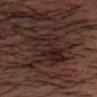<case>
  <biopsy_status>not biopsied; imaged during a skin examination</biopsy_status>
  <lighting>cross-polarized</lighting>
  <lesion_size>
    <long_diameter_mm_approx>6.0</long_diameter_mm_approx>
  </lesion_size>
  <patient>
    <sex>male</sex>
    <age_approx>40</age_approx>
  </patient>
  <site>head or neck</site>
  <image>
    <source>total-body photography crop</source>
    <field_of_view_mm>15</field_of_view_mm>
  </image>
</case>